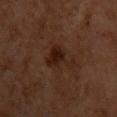Findings:
- workup · catalogued during a skin exam; not biopsied
- patient · male, in their 60s
- illumination · cross-polarized illumination
- site · the chest
- automated lesion analysis · an outline eccentricity of about 0.75 (0 = round, 1 = elongated) and a shape-asymmetry score of about 0.5 (0 = symmetric); an automated nevus-likeness rating near 80 out of 100 and a detector confidence of about 100 out of 100 that the crop contains a lesion
- acquisition · 15 mm crop, total-body photography